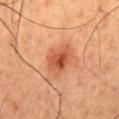Notes:
• workup: no biopsy performed (imaged during a skin exam)
• site: the mid back
• automated lesion analysis: a shape eccentricity near 0.65; a nevus-likeness score of about 85/100 and a lesion-detection confidence of about 100/100
• subject: male, aged 58 to 62
• image source: 15 mm crop, total-body photography
• lighting: cross-polarized illumination